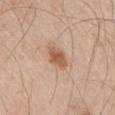biopsy_status: not biopsied; imaged during a skin examination
lighting: white-light
patient:
  sex: male
  age_approx: 60
site: right thigh
image:
  source: total-body photography crop
  field_of_view_mm: 15
lesion_size:
  long_diameter_mm_approx: 3.0